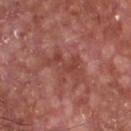Clinical impression: This lesion was catalogued during total-body skin photography and was not selected for biopsy. Background: Longest diameter approximately 5 mm. This image is a 15 mm lesion crop taken from a total-body photograph. From the chest. Automated image analysis of the tile measured a lesion color around L≈44 a*≈28 b*≈27 in CIELAB, roughly 7 lightness units darker than nearby skin, and a normalized border contrast of about 5.5. It also reported a nevus-likeness score of about 0/100 and a detector confidence of about 90 out of 100 that the crop contains a lesion. A male patient roughly 65 years of age.